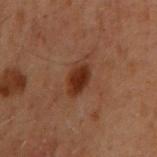{"biopsy_status": "not biopsied; imaged during a skin examination", "image": {"source": "total-body photography crop", "field_of_view_mm": 15}, "lesion_size": {"long_diameter_mm_approx": 3.5}, "patient": {"sex": "male", "age_approx": 60}, "site": "right upper arm"}